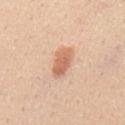Impression: The lesion was tiled from a total-body skin photograph and was not biopsied. Context: The lesion-visualizer software estimated border irregularity of about 3 on a 0–10 scale, a color-variation rating of about 3/10, and radial color variation of about 1. The tile uses white-light illumination. A male patient, roughly 30 years of age. Located on the mid back. Measured at roughly 4 mm in maximum diameter. A 15 mm close-up extracted from a 3D total-body photography capture.